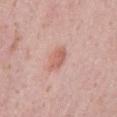site=the left lower leg; imaging modality=~15 mm tile from a whole-body skin photo; patient=female, aged around 40; size=≈3 mm; lighting=white-light.